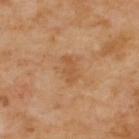Case summary:
• biopsy status · no biopsy performed (imaged during a skin exam)
• imaging modality · 15 mm crop, total-body photography
• image-analysis metrics · a footprint of about 4.5 mm²; an average lesion color of about L≈55 a*≈22 b*≈39 (CIELAB) and a normalized lesion–skin contrast near 5.5; a classifier nevus-likeness of about 0/100 and a lesion-detection confidence of about 100/100
• body site · the upper back
• tile lighting · cross-polarized illumination
• diameter · about 3 mm